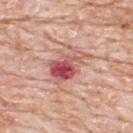| feature | finding |
|---|---|
| notes | total-body-photography surveillance lesion; no biopsy |
| lesion diameter | ≈5 mm |
| subject | male, aged 78–82 |
| imaging modality | 15 mm crop, total-body photography |
| location | the upper back |
| tile lighting | white-light |